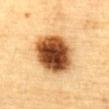Imaged during a routine full-body skin examination; the lesion was not biopsied and no histopathology is available.
Automated tile analysis of the lesion measured a nevus-likeness score of about 100/100 and lesion-presence confidence of about 100/100.
Located on the abdomen.
A male subject, in their mid- to late 80s or older.
A lesion tile, about 15 mm wide, cut from a 3D total-body photograph.